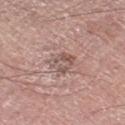* subject · male, aged approximately 75
* body site · the left thigh
* imaging modality · ~15 mm crop, total-body skin-cancer survey
* diameter · about 3 mm
* lighting · white-light